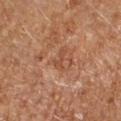Clinical impression:
No biopsy was performed on this lesion — it was imaged during a full skin examination and was not determined to be concerning.
Image and clinical context:
On the right forearm. The tile uses cross-polarized illumination. The subject is a female aged around 65. A roughly 15 mm field-of-view crop from a total-body skin photograph.